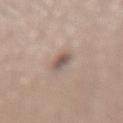No biopsy was performed on this lesion — it was imaged during a full skin examination and was not determined to be concerning.